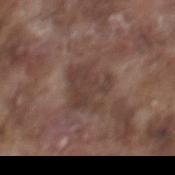A male subject in their mid- to late 70s. The lesion is on the mid back. The tile uses white-light illumination. A region of skin cropped from a whole-body photographic capture, roughly 15 mm wide. Measured at roughly 4.5 mm in maximum diameter.